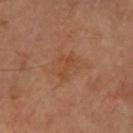Q: Is there a histopathology result?
A: total-body-photography surveillance lesion; no biopsy
Q: Who is the patient?
A: female, in their mid- to late 60s
Q: How was this image acquired?
A: ~15 mm tile from a whole-body skin photo
Q: Automated lesion metrics?
A: a lesion color around L≈47 a*≈24 b*≈35 in CIELAB and a lesion-to-skin contrast of about 5 (normalized; higher = more distinct); a nevus-likeness score of about 0/100 and lesion-presence confidence of about 100/100
Q: Lesion size?
A: ≈3 mm
Q: How was the tile lit?
A: cross-polarized illumination
Q: Lesion location?
A: the left forearm